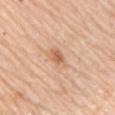Clinical impression: The lesion was tiled from a total-body skin photograph and was not biopsied. Context: Imaged with white-light lighting. Located on the arm. A roughly 15 mm field-of-view crop from a total-body skin photograph. The subject is a female about 65 years old. An algorithmic analysis of the crop reported a lesion area of about 4 mm² and two-axis asymmetry of about 0.3. It also reported a within-lesion color-variation index near 3.5/10 and radial color variation of about 1.